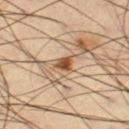notes = catalogued during a skin exam; not biopsied | subject = male, roughly 65 years of age | automated lesion analysis = an average lesion color of about L≈50 a*≈20 b*≈35 (CIELAB) and roughly 15 lightness units darker than nearby skin; border irregularity of about 3 on a 0–10 scale, a color-variation rating of about 3/10, and peripheral color asymmetry of about 1; a classifier nevus-likeness of about 85/100 and a lesion-detection confidence of about 100/100 | lesion size = about 2 mm | image source = total-body-photography crop, ~15 mm field of view | site = the left thigh.